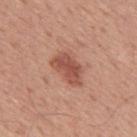Case summary:
* workup: total-body-photography surveillance lesion; no biopsy
* anatomic site: the mid back
* image: ~15 mm tile from a whole-body skin photo
* lighting: white-light illumination
* subject: male, roughly 50 years of age
* automated metrics: a lesion area of about 8.5 mm² and an outline eccentricity of about 0.85 (0 = round, 1 = elongated); a lesion–skin lightness drop of about 11 and a normalized border contrast of about 7.5; border irregularity of about 3 on a 0–10 scale and a peripheral color-asymmetry measure near 1; a classifier nevus-likeness of about 75/100 and a lesion-detection confidence of about 100/100
* lesion diameter: ≈4.5 mm